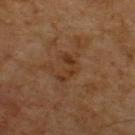Impression: This lesion was catalogued during total-body skin photography and was not selected for biopsy.